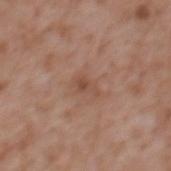This lesion was catalogued during total-body skin photography and was not selected for biopsy. Measured at roughly 3 mm in maximum diameter. Imaged with white-light lighting. This image is a 15 mm lesion crop taken from a total-body photograph. Located on the mid back. The subject is a male approximately 45 years of age.